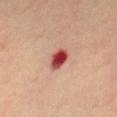This image is a 15 mm lesion crop taken from a total-body photograph.
Measured at roughly 3 mm in maximum diameter.
Located on the left leg.
The subject is a female in their 60s.
Imaged with cross-polarized lighting.